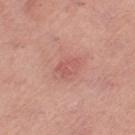Assessment: The lesion was tiled from a total-body skin photograph and was not biopsied. Acquisition and patient details: Located on the right thigh. A 15 mm close-up extracted from a 3D total-body photography capture. The tile uses white-light illumination. Longest diameter approximately 3 mm. A female subject, roughly 65 years of age.